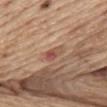<lesion>
<biopsy_status>not biopsied; imaged during a skin examination</biopsy_status>
<site>abdomen</site>
<automated_metrics>
  <area_mm2_approx>3.5</area_mm2_approx>
  <shape_asymmetry>0.25</shape_asymmetry>
  <cielab_L>52</cielab_L>
  <cielab_a>24</cielab_a>
  <cielab_b>28</cielab_b>
  <vs_skin_darker_L>9.0</vs_skin_darker_L>
  <vs_skin_contrast_norm>6.5</vs_skin_contrast_norm>
  <border_irregularity_0_10>2.5</border_irregularity_0_10>
  <color_variation_0_10>10.0</color_variation_0_10>
  <peripheral_color_asymmetry>3.5</peripheral_color_asymmetry>
</automated_metrics>
<image>
  <source>total-body photography crop</source>
  <field_of_view_mm>15</field_of_view_mm>
</image>
<lesion_size>
  <long_diameter_mm_approx>2.5</long_diameter_mm_approx>
</lesion_size>
<lighting>white-light</lighting>
<patient>
  <sex>male</sex>
  <age_approx>70</age_approx>
</patient>
</lesion>